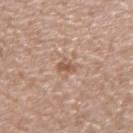The lesion was tiled from a total-body skin photograph and was not biopsied.
A male patient aged around 40.
A 15 mm close-up tile from a total-body photography series done for melanoma screening.
Located on the left forearm.